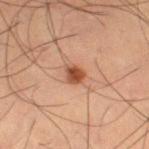notes = imaged on a skin check; not biopsied | image source = ~15 mm tile from a whole-body skin photo | location = the leg | subject = male, about 65 years old.